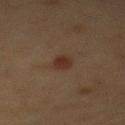Impression:
Recorded during total-body skin imaging; not selected for excision or biopsy.
Context:
An algorithmic analysis of the crop reported a lesion color around L≈26 a*≈16 b*≈21 in CIELAB and roughly 7 lightness units darker than nearby skin. The analysis additionally found a border-irregularity index near 2.5/10 and peripheral color asymmetry of about 0.5. And it measured a nevus-likeness score of about 95/100. Captured under cross-polarized illumination. A region of skin cropped from a whole-body photographic capture, roughly 15 mm wide. Measured at roughly 3 mm in maximum diameter. On the mid back. A female subject about 40 years old.